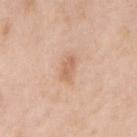  biopsy_status: not biopsied; imaged during a skin examination
  image:
    source: total-body photography crop
    field_of_view_mm: 15
  site: right upper arm
  lesion_size:
    long_diameter_mm_approx: 2.5
  patient:
    sex: female
    age_approx: 40